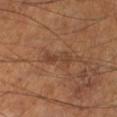Image and clinical context: A roughly 15 mm field-of-view crop from a total-body skin photograph. The lesion-visualizer software estimated an automated nevus-likeness rating near 0 out of 100 and a detector confidence of about 90 out of 100 that the crop contains a lesion. Captured under cross-polarized illumination. On the leg. The recorded lesion diameter is about 4 mm. A male patient roughly 65 years of age.